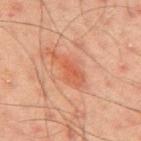notes = imaged on a skin check; not biopsied | lesion diameter = ~5 mm (longest diameter) | imaging modality = total-body-photography crop, ~15 mm field of view | tile lighting = cross-polarized | body site = the mid back | patient = male, aged around 45 | TBP lesion metrics = a border-irregularity index near 5.5/10 and internal color variation of about 2.5 on a 0–10 scale; a lesion-detection confidence of about 100/100.